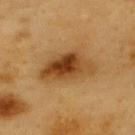Notes:
* follow-up: no biopsy performed (imaged during a skin exam)
* image source: 15 mm crop, total-body photography
* subject: male, approximately 60 years of age
* anatomic site: the upper back